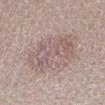{"lighting": "white-light", "patient": {"sex": "female", "age_approx": 45}, "image": {"source": "total-body photography crop", "field_of_view_mm": 15}, "site": "leg"}